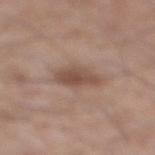This lesion was catalogued during total-body skin photography and was not selected for biopsy.
Longest diameter approximately 4 mm.
Located on the leg.
A male subject, aged 53–57.
The tile uses white-light illumination.
Cropped from a total-body skin-imaging series; the visible field is about 15 mm.